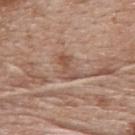| key | value |
|---|---|
| notes | catalogued during a skin exam; not biopsied |
| image-analysis metrics | an area of roughly 5 mm², an eccentricity of roughly 0.9, and two-axis asymmetry of about 0.3 |
| image source | total-body-photography crop, ~15 mm field of view |
| lesion size | ≈3.5 mm |
| patient | female, in their 60s |
| tile lighting | white-light illumination |
| body site | the upper back |